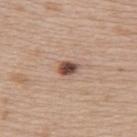Clinical impression:
Imaged during a routine full-body skin examination; the lesion was not biopsied and no histopathology is available.
Clinical summary:
The subject is a female aged 48 to 52. The lesion's longest dimension is about 2.5 mm. A region of skin cropped from a whole-body photographic capture, roughly 15 mm wide. Captured under white-light illumination. The lesion is on the upper back.